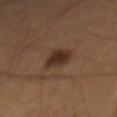follow-up: catalogued during a skin exam; not biopsied | subject: male, aged around 65 | tile lighting: cross-polarized illumination | size: ≈3.5 mm | anatomic site: the mid back | acquisition: total-body-photography crop, ~15 mm field of view.